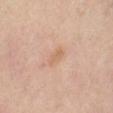| field | value |
|---|---|
| biopsy status | no biopsy performed (imaged during a skin exam) |
| body site | the right thigh |
| acquisition | ~15 mm tile from a whole-body skin photo |
| illumination | cross-polarized |
| size | about 2.5 mm |
| patient | female, aged approximately 50 |